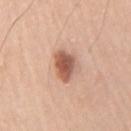* patient · male, aged around 70
* image source · total-body-photography crop, ~15 mm field of view
* body site · the right upper arm
* illumination · white-light illumination